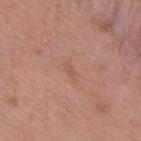workup — catalogued during a skin exam; not biopsied | location — the upper back | acquisition — ~15 mm tile from a whole-body skin photo | tile lighting — white-light illumination | patient — male, aged 48–52.